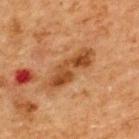| field | value |
|---|---|
| biopsy status | no biopsy performed (imaged during a skin exam) |
| lighting | cross-polarized |
| acquisition | 15 mm crop, total-body photography |
| patient | male, aged 63–67 |
| body site | the back |
| size | about 7 mm |
| automated metrics | an area of roughly 12 mm², a shape eccentricity near 0.95, and a symmetry-axis asymmetry near 0.25; a mean CIELAB color near L≈40 a*≈21 b*≈34, a lesion–skin lightness drop of about 10, and a normalized border contrast of about 8.5 |